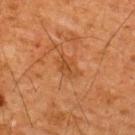| field | value |
|---|---|
| notes | no biopsy performed (imaged during a skin exam) |
| image-analysis metrics | a footprint of about 4.5 mm² and an eccentricity of roughly 0.75; border irregularity of about 2.5 on a 0–10 scale, a within-lesion color-variation index near 2.5/10, and radial color variation of about 1; a nevus-likeness score of about 0/100 and a detector confidence of about 100 out of 100 that the crop contains a lesion |
| tile lighting | cross-polarized illumination |
| image | ~15 mm tile from a whole-body skin photo |
| diameter | ~3 mm (longest diameter) |
| patient | male, aged 63 to 67 |
| site | the back |